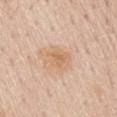This lesion was catalogued during total-body skin photography and was not selected for biopsy. Measured at roughly 3 mm in maximum diameter. A male subject, aged around 60. The lesion is located on the mid back. A region of skin cropped from a whole-body photographic capture, roughly 15 mm wide. Imaged with white-light lighting.